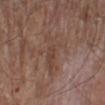follow-up = imaged on a skin check; not biopsied
body site = the left lower leg
lesion size = ~5.5 mm (longest diameter)
imaging modality = ~15 mm tile from a whole-body skin photo
illumination = white-light illumination
subject = male, aged 78–82